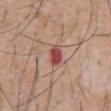Background: From the abdomen. Cropped from a whole-body photographic skin survey; the tile spans about 15 mm. Measured at roughly 2.5 mm in maximum diameter. The subject is a male about 55 years old. Captured under white-light illumination. The total-body-photography lesion software estimated an average lesion color of about L≈48 a*≈31 b*≈24 (CIELAB), a lesion–skin lightness drop of about 14, and a normalized lesion–skin contrast near 10. The software also gave border irregularity of about 1.5 on a 0–10 scale, internal color variation of about 3.5 on a 0–10 scale, and radial color variation of about 1. The software also gave an automated nevus-likeness rating near 0 out of 100 and lesion-presence confidence of about 100/100.